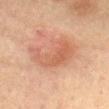biopsy status=total-body-photography surveillance lesion; no biopsy | subject=male, aged 58–62 | anatomic site=the mid back | tile lighting=cross-polarized | lesion size=about 7 mm | automated metrics=a footprint of about 18 mm² and an eccentricity of roughly 0.85 | image=~15 mm crop, total-body skin-cancer survey.